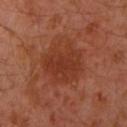biopsy status = catalogued during a skin exam; not biopsied
acquisition = ~15 mm tile from a whole-body skin photo
subject = male, aged 28 to 32
location = the arm